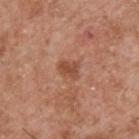Assessment:
The lesion was photographed on a routine skin check and not biopsied; there is no pathology result.
Context:
A 15 mm crop from a total-body photograph taken for skin-cancer surveillance. From the upper back. Measured at roughly 2.5 mm in maximum diameter. A male subject, aged around 55.